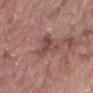biopsy status = catalogued during a skin exam; not biopsied | lesion size = ≈4 mm | illumination = white-light illumination | image-analysis metrics = internal color variation of about 3 on a 0–10 scale and radial color variation of about 1 | patient = female, aged 68 to 72 | acquisition = ~15 mm tile from a whole-body skin photo | site = the right lower leg.